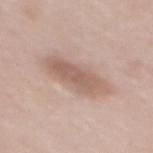  biopsy_status: not biopsied; imaged during a skin examination
  automated_metrics:
    cielab_L: 60
    cielab_a: 17
    cielab_b: 26
    vs_skin_darker_L: 10.0
    vs_skin_contrast_norm: 6.5
  site: upper back
  lighting: white-light
  image:
    source: total-body photography crop
    field_of_view_mm: 15
  patient:
    sex: female
    age_approx: 50